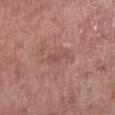- biopsy status: catalogued during a skin exam; not biopsied
- lesion diameter: about 2.5 mm
- TBP lesion metrics: a lesion color around L≈50 a*≈22 b*≈24 in CIELAB, a lesion–skin lightness drop of about 6, and a normalized border contrast of about 5; border irregularity of about 5.5 on a 0–10 scale, internal color variation of about 0 on a 0–10 scale, and radial color variation of about 0
- lighting: white-light illumination
- subject: male, aged around 55
- anatomic site: the leg
- imaging modality: ~15 mm crop, total-body skin-cancer survey